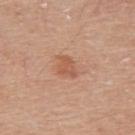biopsy status: catalogued during a skin exam; not biopsied | patient: male, aged 78–82 | body site: the upper back | size: ≈3 mm | imaging modality: 15 mm crop, total-body photography | tile lighting: white-light.